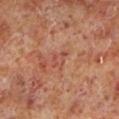follow-up — no biopsy performed (imaged during a skin exam)
acquisition — ~15 mm tile from a whole-body skin photo
illumination — cross-polarized illumination
anatomic site — the left lower leg
subject — male, roughly 60 years of age